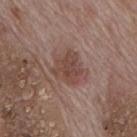follow-up — no biopsy performed (imaged during a skin exam)
subject — male, aged around 70
tile lighting — white-light
anatomic site — the mid back
image source — ~15 mm tile from a whole-body skin photo